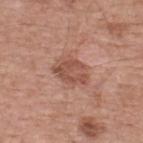| key | value |
|---|---|
| biopsy status | catalogued during a skin exam; not biopsied |
| site | the back |
| image | 15 mm crop, total-body photography |
| diameter | ~4.5 mm (longest diameter) |
| patient | male, aged 53 to 57 |
| TBP lesion metrics | a lesion color around L≈52 a*≈23 b*≈28 in CIELAB and about 9 CIELAB-L* units darker than the surrounding skin; a border-irregularity index near 2/10 and a color-variation rating of about 4/10; an automated nevus-likeness rating near 10 out of 100 and lesion-presence confidence of about 100/100 |